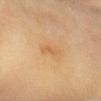  biopsy_status: not biopsied; imaged during a skin examination
  image:
    source: total-body photography crop
    field_of_view_mm: 15
  site: arm
  patient:
    sex: female
    age_approx: 60
  lesion_size:
    long_diameter_mm_approx: 3.0
  lighting: cross-polarized